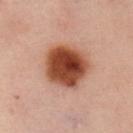Captured during whole-body skin photography for melanoma surveillance; the lesion was not biopsied.
A 15 mm close-up extracted from a 3D total-body photography capture.
Located on the chest.
The patient is a female in their mid- to late 50s.
The tile uses cross-polarized illumination.